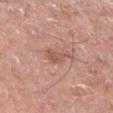Impression: This lesion was catalogued during total-body skin photography and was not selected for biopsy. Background: Cropped from a total-body skin-imaging series; the visible field is about 15 mm. On the left lower leg. Imaged with white-light lighting. The subject is a male roughly 55 years of age. Longest diameter approximately 3.5 mm.